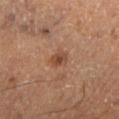Clinical impression: Imaged during a routine full-body skin examination; the lesion was not biopsied and no histopathology is available. Clinical summary: On the left lower leg. Longest diameter approximately 2 mm. A male subject, about 60 years old. Automated image analysis of the tile measured an average lesion color of about L≈40 a*≈21 b*≈29 (CIELAB) and a lesion-to-skin contrast of about 7.5 (normalized; higher = more distinct). The analysis additionally found internal color variation of about 3 on a 0–10 scale and radial color variation of about 1. The software also gave an automated nevus-likeness rating near 65 out of 100 and a lesion-detection confidence of about 100/100. The tile uses cross-polarized illumination. A 15 mm close-up extracted from a 3D total-body photography capture.